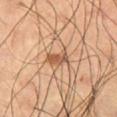This lesion was catalogued during total-body skin photography and was not selected for biopsy. The lesion is located on the left thigh. Imaged with cross-polarized lighting. Cropped from a total-body skin-imaging series; the visible field is about 15 mm. The lesion-visualizer software estimated a detector confidence of about 100 out of 100 that the crop contains a lesion. The recorded lesion diameter is about 3 mm. The subject is a male aged 58 to 62.